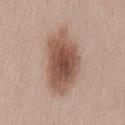workup — catalogued during a skin exam; not biopsied | illumination — white-light | automated lesion analysis — a footprint of about 27 mm², an eccentricity of roughly 0.8, and two-axis asymmetry of about 0.2; a border-irregularity index near 2.5/10, a within-lesion color-variation index near 5.5/10, and a peripheral color-asymmetry measure near 1.5 | location — the lower back | diameter — ~8 mm (longest diameter) | acquisition — total-body-photography crop, ~15 mm field of view | patient — male, aged 53 to 57.